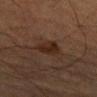automated metrics: an outline eccentricity of about 0.7 (0 = round, 1 = elongated) and two-axis asymmetry of about 0.3; about 7 CIELAB-L* units darker than the surrounding skin and a normalized border contrast of about 8.5; a detector confidence of about 100 out of 100 that the crop contains a lesion
subject: male, approximately 85 years of age or older
lesion size: about 3 mm
site: the left forearm
illumination: cross-polarized illumination
acquisition: total-body-photography crop, ~15 mm field of view